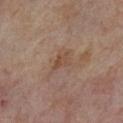Imaged during a routine full-body skin examination; the lesion was not biopsied and no histopathology is available. A 15 mm close-up extracted from a 3D total-body photography capture. A female subject about 55 years old. The recorded lesion diameter is about 2.5 mm. Captured under cross-polarized illumination. From the right thigh.